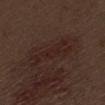Impression: This lesion was catalogued during total-body skin photography and was not selected for biopsy. Background: An algorithmic analysis of the crop reported a footprint of about 8 mm² and a shape eccentricity near 0.95. The analysis additionally found an average lesion color of about L≈22 a*≈17 b*≈19 (CIELAB), about 5 CIELAB-L* units darker than the surrounding skin, and a normalized border contrast of about 5.5. It also reported a border-irregularity rating of about 8.5/10, a within-lesion color-variation index near 1.5/10, and peripheral color asymmetry of about 0.5. The subject is a male aged 68–72. About 5.5 mm across. A close-up tile cropped from a whole-body skin photograph, about 15 mm across. On the left thigh.